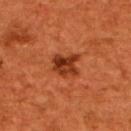{"biopsy_status": "not biopsied; imaged during a skin examination", "site": "upper back", "image": {"source": "total-body photography crop", "field_of_view_mm": 15}, "patient": {"sex": "male", "age_approx": 45}, "lesion_size": {"long_diameter_mm_approx": 3.0}, "automated_metrics": {"area_mm2_approx": 7.0, "eccentricity": 0.55, "shape_asymmetry": 0.25, "border_irregularity_0_10": 3.0, "color_variation_0_10": 5.5, "nevus_likeness_0_100": 100, "lesion_detection_confidence_0_100": 100}}